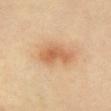Clinical impression: Imaged during a routine full-body skin examination; the lesion was not biopsied and no histopathology is available.